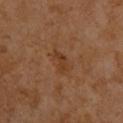Impression: Recorded during total-body skin imaging; not selected for excision or biopsy. Image and clinical context: Automated image analysis of the tile measured a lesion area of about 3.5 mm² and an outline eccentricity of about 0.85 (0 = round, 1 = elongated). The software also gave a mean CIELAB color near L≈35 a*≈20 b*≈32, a lesion–skin lightness drop of about 6, and a normalized lesion–skin contrast near 6.5. The analysis additionally found a classifier nevus-likeness of about 5/100 and a detector confidence of about 100 out of 100 that the crop contains a lesion. A male subject approximately 60 years of age. From the back. Cropped from a total-body skin-imaging series; the visible field is about 15 mm.